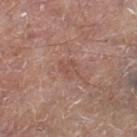This lesion was catalogued during total-body skin photography and was not selected for biopsy.
The total-body-photography lesion software estimated a shape eccentricity near 0.65 and two-axis asymmetry of about 0.25. It also reported a mean CIELAB color near L≈51 a*≈21 b*≈26, roughly 6 lightness units darker than nearby skin, and a normalized border contrast of about 4.5.
This is a white-light tile.
A 15 mm close-up tile from a total-body photography series done for melanoma screening.
The patient is a male roughly 65 years of age.
The lesion is on the left thigh.
Longest diameter approximately 2.5 mm.